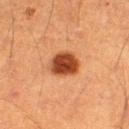Acquisition and patient details:
The lesion is located on the right thigh. A male subject, about 60 years old. Captured under cross-polarized illumination. The total-body-photography lesion software estimated a border-irregularity index near 1.5/10 and peripheral color asymmetry of about 1. And it measured a classifier nevus-likeness of about 100/100. A close-up tile cropped from a whole-body skin photograph, about 15 mm across.